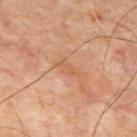biopsy status=imaged on a skin check; not biopsied
tile lighting=cross-polarized illumination
patient=male, about 65 years old
body site=the mid back
image source=15 mm crop, total-body photography
lesion diameter=about 3 mm